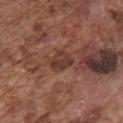Impression:
No biopsy was performed on this lesion — it was imaged during a full skin examination and was not determined to be concerning.
Clinical summary:
Cropped from a whole-body photographic skin survey; the tile spans about 15 mm. The lesion is located on the front of the torso. Captured under white-light illumination. The patient is a male aged 73 to 77. Measured at roughly 3 mm in maximum diameter.